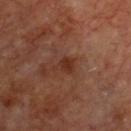  biopsy_status: not biopsied; imaged during a skin examination
  lighting: cross-polarized
  automated_metrics:
    area_mm2_approx: 4.0
    eccentricity: 0.7
    shape_asymmetry: 0.35
    border_irregularity_0_10: 3.5
    color_variation_0_10: 2.0
    peripheral_color_asymmetry: 0.5
  patient:
    sex: male
    age_approx: 65
  site: chest
  image:
    source: total-body photography crop
    field_of_view_mm: 15
  lesion_size:
    long_diameter_mm_approx: 2.5